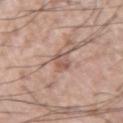Impression: No biopsy was performed on this lesion — it was imaged during a full skin examination and was not determined to be concerning. Background: The lesion-visualizer software estimated a lesion-detection confidence of about 90/100. A 15 mm crop from a total-body photograph taken for skin-cancer surveillance. A male patient, aged approximately 55. Approximately 3 mm at its widest. From the arm.